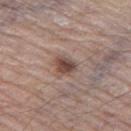{"biopsy_status": "not biopsied; imaged during a skin examination", "lesion_size": {"long_diameter_mm_approx": 2.5}, "patient": {"sex": "male", "age_approx": 75}, "automated_metrics": {"border_irregularity_0_10": 2.0, "color_variation_0_10": 3.5, "peripheral_color_asymmetry": 1.0, "nevus_likeness_0_100": 85, "lesion_detection_confidence_0_100": 100}, "site": "left thigh", "image": {"source": "total-body photography crop", "field_of_view_mm": 15}, "lighting": "white-light"}